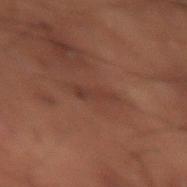Captured during whole-body skin photography for melanoma surveillance; the lesion was not biopsied.
A 15 mm crop from a total-body photograph taken for skin-cancer surveillance.
The lesion is on the right thigh.
The patient is a male approximately 50 years of age.